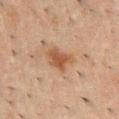biopsy_status: not biopsied; imaged during a skin examination
patient:
  sex: male
  age_approx: 50
image:
  source: total-body photography crop
  field_of_view_mm: 15
site: chest
automated_metrics:
  area_mm2_approx: 6.5
  eccentricity: 0.65
  shape_asymmetry: 0.4
  cielab_L: 41
  cielab_a: 18
  cielab_b: 28
  vs_skin_darker_L: 8.0
  vs_skin_contrast_norm: 8.0
  lesion_detection_confidence_0_100: 100